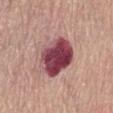follow-up: imaged on a skin check; not biopsied
automated metrics: an area of roughly 18 mm², a shape eccentricity near 0.5, and a shape-asymmetry score of about 0.1 (0 = symmetric); an average lesion color of about L≈44 a*≈31 b*≈17 (CIELAB), roughly 21 lightness units darker than nearby skin, and a lesion-to-skin contrast of about 15.5 (normalized; higher = more distinct)
imaging modality: ~15 mm crop, total-body skin-cancer survey
body site: the front of the torso
subject: female, roughly 65 years of age